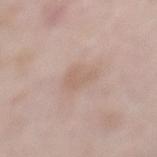Q: How was this image acquired?
A: ~15 mm crop, total-body skin-cancer survey
Q: What are the patient's age and sex?
A: male, aged approximately 55
Q: What is the lesion's diameter?
A: ≈3 mm
Q: Lesion location?
A: the mid back
Q: What did automated image analysis measure?
A: an average lesion color of about L≈61 a*≈16 b*≈26 (CIELAB), roughly 6 lightness units darker than nearby skin, and a normalized border contrast of about 5; a nevus-likeness score of about 0/100 and a detector confidence of about 100 out of 100 that the crop contains a lesion